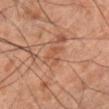notes — imaged on a skin check; not biopsied | diameter — ~2.5 mm (longest diameter) | location — the right lower leg | tile lighting — cross-polarized illumination | subject — male, aged 58–62 | imaging modality — 15 mm crop, total-body photography | TBP lesion metrics — an area of roughly 3.5 mm² and a shape eccentricity near 0.65; peripheral color asymmetry of about 0.